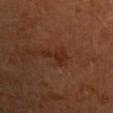Part of a total-body skin-imaging series; this lesion was reviewed on a skin check and was not flagged for biopsy. The lesion is located on the right upper arm. A male patient aged 53 to 57. The recorded lesion diameter is about 3.5 mm. This is a cross-polarized tile. Cropped from a whole-body photographic skin survey; the tile spans about 15 mm.